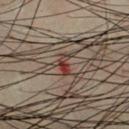Q: Is there a histopathology result?
A: no biopsy performed (imaged during a skin exam)
Q: What is the anatomic site?
A: the chest
Q: Lesion size?
A: ≈2.5 mm
Q: What lighting was used for the tile?
A: cross-polarized
Q: What are the patient's age and sex?
A: male, roughly 50 years of age
Q: What kind of image is this?
A: 15 mm crop, total-body photography